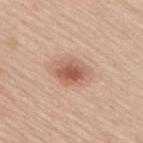Q: Was this lesion biopsied?
A: catalogued during a skin exam; not biopsied
Q: What is the imaging modality?
A: total-body-photography crop, ~15 mm field of view
Q: What is the anatomic site?
A: the back
Q: How was the tile lit?
A: white-light illumination
Q: What are the patient's age and sex?
A: male, in their mid- to late 40s
Q: What did automated image analysis measure?
A: a footprint of about 8.5 mm² and a shape-asymmetry score of about 0.15 (0 = symmetric); a mean CIELAB color near L≈58 a*≈22 b*≈30 and roughly 12 lightness units darker than nearby skin; an automated nevus-likeness rating near 90 out of 100 and a detector confidence of about 100 out of 100 that the crop contains a lesion
Q: Lesion size?
A: ≈4 mm